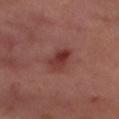workup: imaged on a skin check; not biopsied | patient: female, in their mid- to late 50s | lesion size: ~3.5 mm (longest diameter) | image source: total-body-photography crop, ~15 mm field of view | anatomic site: the right thigh.